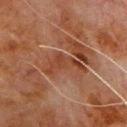Q: Was this lesion biopsied?
A: imaged on a skin check; not biopsied
Q: Where on the body is the lesion?
A: the chest
Q: Illumination type?
A: cross-polarized illumination
Q: What is the lesion's diameter?
A: ~5 mm (longest diameter)
Q: Patient demographics?
A: male, aged around 80
Q: What is the imaging modality?
A: ~15 mm tile from a whole-body skin photo